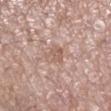Impression: The lesion was tiled from a total-body skin photograph and was not biopsied. Acquisition and patient details: A female patient in their mid-70s. A close-up tile cropped from a whole-body skin photograph, about 15 mm across. The lesion is on the right lower leg. About 3 mm across. Captured under white-light illumination. The total-body-photography lesion software estimated an area of roughly 5 mm² and a shape eccentricity near 0.5.